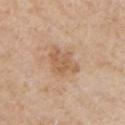notes: catalogued during a skin exam; not biopsied
site: the chest
lighting: white-light
image: ~15 mm crop, total-body skin-cancer survey
automated lesion analysis: a lesion area of about 8 mm², an outline eccentricity of about 0.7 (0 = round, 1 = elongated), and two-axis asymmetry of about 0.35; a border-irregularity index near 4.5/10, a color-variation rating of about 2.5/10, and radial color variation of about 1
diameter: ~4 mm (longest diameter)
patient: male, approximately 65 years of age